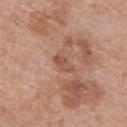Assessment:
No biopsy was performed on this lesion — it was imaged during a full skin examination and was not determined to be concerning.
Context:
A 15 mm close-up extracted from a 3D total-body photography capture. A male subject about 70 years old. This is a white-light tile. From the upper back. About 3 mm across.